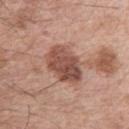follow-up — catalogued during a skin exam; not biopsied
image — ~15 mm crop, total-body skin-cancer survey
patient — male, aged 63–67
body site — the right upper arm